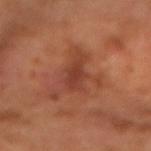  biopsy_status: not biopsied; imaged during a skin examination
  lighting: cross-polarized
  image:
    source: total-body photography crop
    field_of_view_mm: 15
  patient:
    sex: male
    age_approx: 65
  automated_metrics:
    vs_skin_darker_L: 8.0
    vs_skin_contrast_norm: 6.5